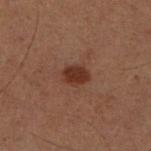The lesion is on the right lower leg.
Longest diameter approximately 3 mm.
A male subject, about 60 years old.
The tile uses cross-polarized illumination.
A close-up tile cropped from a whole-body skin photograph, about 15 mm across.